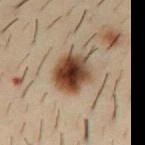Clinical impression:
The lesion was tiled from a total-body skin photograph and was not biopsied.
Clinical summary:
A male subject, about 30 years old. The lesion is located on the abdomen. The tile uses cross-polarized illumination. A 15 mm close-up extracted from a 3D total-body photography capture.